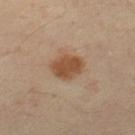biopsy_status: not biopsied; imaged during a skin examination
image:
  source: total-body photography crop
  field_of_view_mm: 15
lighting: cross-polarized
site: right leg
lesion_size:
  long_diameter_mm_approx: 4.0
patient:
  sex: female
  age_approx: 40